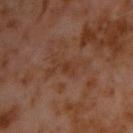Notes:
– notes · no biopsy performed (imaged during a skin exam)
– tile lighting · cross-polarized
– size · ~3 mm (longest diameter)
– anatomic site · the upper back
– imaging modality · ~15 mm crop, total-body skin-cancer survey
– subject · male, in their 60s